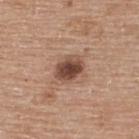{
  "biopsy_status": "not biopsied; imaged during a skin examination",
  "automated_metrics": {
    "eccentricity": 0.7,
    "shape_asymmetry": 0.15
  },
  "image": {
    "source": "total-body photography crop",
    "field_of_view_mm": 15
  },
  "site": "upper back",
  "patient": {
    "sex": "female",
    "age_approx": 60
  },
  "lighting": "white-light",
  "lesion_size": {
    "long_diameter_mm_approx": 3.5
  }
}